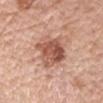Q: Was this lesion biopsied?
A: total-body-photography surveillance lesion; no biopsy
Q: Lesion location?
A: the right upper arm
Q: Who is the patient?
A: female, in their mid-60s
Q: What is the imaging modality?
A: ~15 mm crop, total-body skin-cancer survey